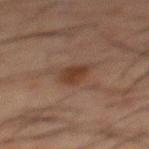– workup · no biopsy performed (imaged during a skin exam)
– subject · male, aged 48–52
– size · ~3 mm (longest diameter)
– body site · the mid back
– image source · 15 mm crop, total-body photography
– lighting · cross-polarized illumination